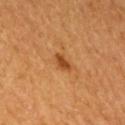Assessment: The lesion was photographed on a routine skin check and not biopsied; there is no pathology result. Acquisition and patient details: A 15 mm crop from a total-body photograph taken for skin-cancer surveillance. The subject is a male about 55 years old. On the arm. Imaged with cross-polarized lighting. Measured at roughly 2.5 mm in maximum diameter.